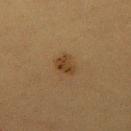Clinical impression: Captured during whole-body skin photography for melanoma surveillance; the lesion was not biopsied. Background: Imaged with cross-polarized lighting. Located on the mid back. The patient is a female in their 40s. A 15 mm close-up tile from a total-body photography series done for melanoma screening. Approximately 3 mm at its widest.